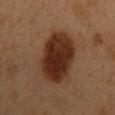{
  "biopsy_status": "not biopsied; imaged during a skin examination",
  "site": "back",
  "patient": {
    "sex": "male",
    "age_approx": 50
  },
  "lighting": "cross-polarized",
  "image": {
    "source": "total-body photography crop",
    "field_of_view_mm": 15
  },
  "lesion_size": {
    "long_diameter_mm_approx": 7.5
  }
}